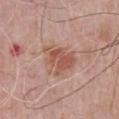• workup · total-body-photography surveillance lesion; no biopsy
• image-analysis metrics · an area of roughly 11 mm², a shape eccentricity near 0.65, and a shape-asymmetry score of about 0.3 (0 = symmetric); an automated nevus-likeness rating near 80 out of 100
• subject · male, aged 63 to 67
• lighting · white-light
• diameter · about 4.5 mm
• site · the chest
• imaging modality · ~15 mm crop, total-body skin-cancer survey